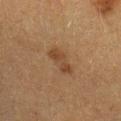Impression:
This lesion was catalogued during total-body skin photography and was not selected for biopsy.
Image and clinical context:
The lesion-visualizer software estimated an average lesion color of about L≈35 a*≈16 b*≈28 (CIELAB), roughly 7 lightness units darker than nearby skin, and a lesion-to-skin contrast of about 6.5 (normalized; higher = more distinct). From the arm. A roughly 15 mm field-of-view crop from a total-body skin photograph. A female subject about 40 years old. Measured at roughly 3.5 mm in maximum diameter.